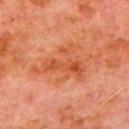* workup · no biopsy performed (imaged during a skin exam)
* automated lesion analysis · an eccentricity of roughly 0.75 and two-axis asymmetry of about 0.6; an average lesion color of about L≈42 a*≈25 b*≈33 (CIELAB) and a lesion-to-skin contrast of about 6 (normalized; higher = more distinct)
* diameter · ≈6.5 mm
* patient · male, roughly 80 years of age
* illumination · cross-polarized illumination
* body site · the left upper arm
* acquisition · 15 mm crop, total-body photography